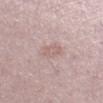<case>
  <biopsy_status>not biopsied; imaged during a skin examination</biopsy_status>
  <image>
    <source>total-body photography crop</source>
    <field_of_view_mm>15</field_of_view_mm>
  </image>
  <site>right lower leg</site>
  <patient>
    <sex>female</sex>
    <age_approx>50</age_approx>
  </patient>
  <lesion_size>
    <long_diameter_mm_approx>2.5</long_diameter_mm_approx>
  </lesion_size>
</case>